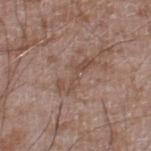workup: imaged on a skin check; not biopsied
lesion diameter: ~5 mm (longest diameter)
imaging modality: ~15 mm tile from a whole-body skin photo
illumination: white-light
anatomic site: the right lower leg
patient: male, aged approximately 60
TBP lesion metrics: an area of roughly 7 mm² and a shape-asymmetry score of about 0.65 (0 = symmetric); an automated nevus-likeness rating near 0 out of 100 and lesion-presence confidence of about 80/100